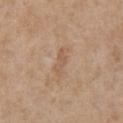This lesion was catalogued during total-body skin photography and was not selected for biopsy. A male patient about 65 years old. A lesion tile, about 15 mm wide, cut from a 3D total-body photograph. On the chest. Imaged with white-light lighting. Measured at roughly 3.5 mm in maximum diameter.